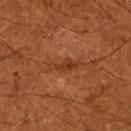Impression:
No biopsy was performed on this lesion — it was imaged during a full skin examination and was not determined to be concerning.
Clinical summary:
A roughly 15 mm field-of-view crop from a total-body skin photograph. Automated image analysis of the tile measured roughly 7 lightness units darker than nearby skin and a lesion-to-skin contrast of about 6.5 (normalized; higher = more distinct). And it measured a border-irregularity index near 4.5/10, internal color variation of about 2 on a 0–10 scale, and peripheral color asymmetry of about 0.5. Longest diameter approximately 4.5 mm. Imaged with cross-polarized lighting. On the leg. The subject is a male approximately 65 years of age.